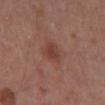Case summary:
- workup: catalogued during a skin exam; not biopsied
- image source: 15 mm crop, total-body photography
- patient: male, approximately 55 years of age
- lesion diameter: about 2.5 mm
- illumination: white-light
- site: the abdomen